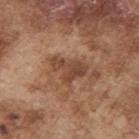| key | value |
|---|---|
| notes | catalogued during a skin exam; not biopsied |
| patient | male, about 75 years old |
| illumination | white-light |
| lesion diameter | about 4.5 mm |
| location | the right upper arm |
| image-analysis metrics | a lesion color around L≈45 a*≈21 b*≈30 in CIELAB, about 10 CIELAB-L* units darker than the surrounding skin, and a normalized border contrast of about 7.5; a border-irregularity index near 5.5/10 and a color-variation rating of about 3/10; a detector confidence of about 100 out of 100 that the crop contains a lesion |
| image source | total-body-photography crop, ~15 mm field of view |